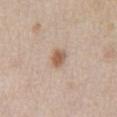{"biopsy_status": "not biopsied; imaged during a skin examination", "image": {"source": "total-body photography crop", "field_of_view_mm": 15}, "patient": {"sex": "male", "age_approx": 65}, "site": "front of the torso", "lighting": "white-light", "lesion_size": {"long_diameter_mm_approx": 2.5}}